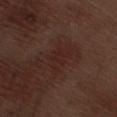Part of a total-body skin-imaging series; this lesion was reviewed on a skin check and was not flagged for biopsy.
A roughly 15 mm field-of-view crop from a total-body skin photograph.
Captured under white-light illumination.
From the right thigh.
A male patient, aged 68–72.
Longest diameter approximately 4 mm.